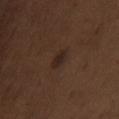Impression:
Recorded during total-body skin imaging; not selected for excision or biopsy.
Image and clinical context:
Captured under white-light illumination. From the chest. About 2.5 mm across. Cropped from a whole-body photographic skin survey; the tile spans about 15 mm. Automated tile analysis of the lesion measured a lesion area of about 3 mm², an eccentricity of roughly 0.85, and a shape-asymmetry score of about 0.2 (0 = symmetric). And it measured about 6 CIELAB-L* units darker than the surrounding skin and a normalized lesion–skin contrast near 8. And it measured a border-irregularity rating of about 2/10, internal color variation of about 1.5 on a 0–10 scale, and peripheral color asymmetry of about 0.5. A male subject approximately 70 years of age.